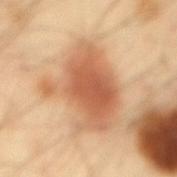Captured during whole-body skin photography for melanoma surveillance; the lesion was not biopsied.
The subject is a male in their 40s.
A roughly 15 mm field-of-view crop from a total-body skin photograph.
Measured at roughly 8 mm in maximum diameter.
Imaged with cross-polarized lighting.
On the back.